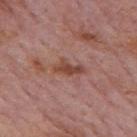Captured during whole-body skin photography for melanoma surveillance; the lesion was not biopsied.
A roughly 15 mm field-of-view crop from a total-body skin photograph.
The patient is a male aged approximately 75.
The lesion is located on the mid back.